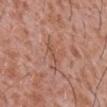{"biopsy_status": "not biopsied; imaged during a skin examination"}